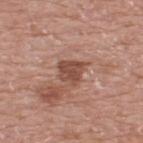The lesion was tiled from a total-body skin photograph and was not biopsied.
Cropped from a total-body skin-imaging series; the visible field is about 15 mm.
An algorithmic analysis of the crop reported a lesion–skin lightness drop of about 11 and a normalized lesion–skin contrast near 8. The software also gave lesion-presence confidence of about 100/100.
Captured under white-light illumination.
A male subject in their mid-70s.
The lesion is on the upper back.
About 3 mm across.